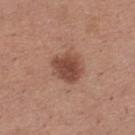workup: no biopsy performed (imaged during a skin exam)
lighting: white-light
automated lesion analysis: an average lesion color of about L≈46 a*≈22 b*≈28 (CIELAB), a lesion–skin lightness drop of about 12, and a normalized lesion–skin contrast near 9; a border-irregularity rating of about 2.5/10 and radial color variation of about 1
patient: female, roughly 30 years of age
location: the left thigh
image source: ~15 mm crop, total-body skin-cancer survey